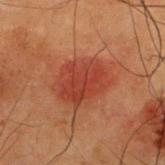The lesion was photographed on a routine skin check and not biopsied; there is no pathology result. This is a cross-polarized tile. The lesion's longest dimension is about 5.5 mm. An algorithmic analysis of the crop reported a detector confidence of about 100 out of 100 that the crop contains a lesion. The lesion is located on the upper back. A 15 mm close-up tile from a total-body photography series done for melanoma screening. The subject is a male in their 50s.